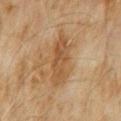biopsy_status: not biopsied; imaged during a skin examination
lesion_size:
  long_diameter_mm_approx: 7.0
image:
  source: total-body photography crop
  field_of_view_mm: 15
patient:
  sex: male
  age_approx: 60
site: front of the torso
lighting: cross-polarized